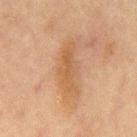{
  "biopsy_status": "not biopsied; imaged during a skin examination",
  "image": {
    "source": "total-body photography crop",
    "field_of_view_mm": 15
  },
  "patient": {
    "sex": "male",
    "age_approx": 65
  },
  "site": "chest"
}